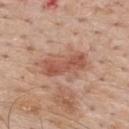<lesion>
  <biopsy_status>not biopsied; imaged during a skin examination</biopsy_status>
  <image>
    <source>total-body photography crop</source>
    <field_of_view_mm>15</field_of_view_mm>
  </image>
  <patient>
    <sex>male</sex>
    <age_approx>60</age_approx>
  </patient>
  <site>upper back</site>
</lesion>